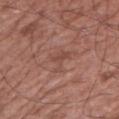notes: catalogued during a skin exam; not biopsied | patient: male, aged around 75 | lesion diameter: ~3.5 mm (longest diameter) | image-analysis metrics: a shape eccentricity near 0.85 and a symmetry-axis asymmetry near 0.6; a mean CIELAB color near L≈47 a*≈22 b*≈25 and a normalized border contrast of about 5; a classifier nevus-likeness of about 0/100 and lesion-presence confidence of about 90/100 | anatomic site: the right upper arm | image: ~15 mm crop, total-body skin-cancer survey | tile lighting: white-light.